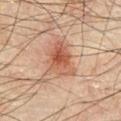<case>
  <biopsy_status>not biopsied; imaged during a skin examination</biopsy_status>
  <image>
    <source>total-body photography crop</source>
    <field_of_view_mm>15</field_of_view_mm>
  </image>
  <site>front of the torso</site>
  <lesion_size>
    <long_diameter_mm_approx>5.0</long_diameter_mm_approx>
  </lesion_size>
  <patient>
    <sex>male</sex>
    <age_approx>70</age_approx>
  </patient>
  <automated_metrics>
    <border_irregularity_0_10>4.0</border_irregularity_0_10>
    <peripheral_color_asymmetry>2.0</peripheral_color_asymmetry>
  </automated_metrics>
</case>